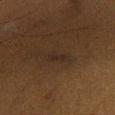– notes · imaged on a skin check; not biopsied
– TBP lesion metrics · an area of roughly 3 mm² and an eccentricity of roughly 0.9; a lesion color around L≈20 a*≈11 b*≈19 in CIELAB, roughly 4 lightness units darker than nearby skin, and a normalized border contrast of about 6; a border-irregularity index near 3.5/10 and a within-lesion color-variation index near 0/10
– location · the right lower leg
– subject · male, roughly 60 years of age
– lighting · cross-polarized illumination
– imaging modality · ~15 mm crop, total-body skin-cancer survey